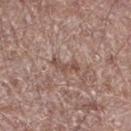Recorded during total-body skin imaging; not selected for excision or biopsy. A male subject aged 68–72. On the right thigh. This image is a 15 mm lesion crop taken from a total-body photograph. Imaged with white-light lighting.